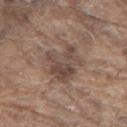workup: imaged on a skin check; not biopsied
size: ~5 mm (longest diameter)
lighting: white-light illumination
anatomic site: the back
patient: male, approximately 80 years of age
image source: total-body-photography crop, ~15 mm field of view